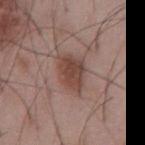| field | value |
|---|---|
| workup | imaged on a skin check; not biopsied |
| subject | male, roughly 55 years of age |
| acquisition | 15 mm crop, total-body photography |
| automated metrics | a footprint of about 9 mm², an outline eccentricity of about 0.7 (0 = round, 1 = elongated), and two-axis asymmetry of about 0.25; peripheral color asymmetry of about 1; a classifier nevus-likeness of about 95/100 and a detector confidence of about 100 out of 100 that the crop contains a lesion |
| illumination | white-light illumination |
| body site | the abdomen |
| lesion size | ≈4 mm |